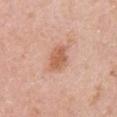patient = female, aged around 30
acquisition = total-body-photography crop, ~15 mm field of view
site = the right upper arm
automated lesion analysis = a border-irregularity rating of about 1.5/10, internal color variation of about 2 on a 0–10 scale, and a peripheral color-asymmetry measure near 0.5; a classifier nevus-likeness of about 75/100 and a detector confidence of about 100 out of 100 that the crop contains a lesion
illumination = white-light